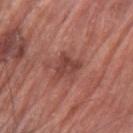Imaged during a routine full-body skin examination; the lesion was not biopsied and no histopathology is available. A male patient approximately 70 years of age. The lesion is located on the arm. A 15 mm close-up tile from a total-body photography series done for melanoma screening.